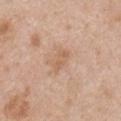Assessment:
Part of a total-body skin-imaging series; this lesion was reviewed on a skin check and was not flagged for biopsy.
Acquisition and patient details:
From the chest. Imaged with white-light lighting. A 15 mm close-up extracted from a 3D total-body photography capture. A male subject, aged approximately 50.